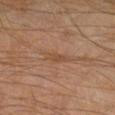Notes:
– body site: the right lower leg
– tile lighting: cross-polarized
– image: ~15 mm tile from a whole-body skin photo
– patient: male, aged 58 to 62